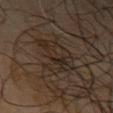No biopsy was performed on this lesion — it was imaged during a full skin examination and was not determined to be concerning.